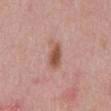Q: Was a biopsy performed?
A: no biopsy performed (imaged during a skin exam)
Q: How was the tile lit?
A: white-light illumination
Q: What is the anatomic site?
A: the abdomen
Q: How was this image acquired?
A: ~15 mm crop, total-body skin-cancer survey
Q: What are the patient's age and sex?
A: male, roughly 50 years of age